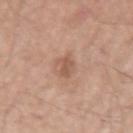Imaged during a routine full-body skin examination; the lesion was not biopsied and no histopathology is available.
A 15 mm crop from a total-body photograph taken for skin-cancer surveillance.
A male patient, aged approximately 55.
From the arm.
An algorithmic analysis of the crop reported a lesion area of about 3.5 mm² and an outline eccentricity of about 0.9 (0 = round, 1 = elongated). The software also gave a border-irregularity rating of about 4/10, a within-lesion color-variation index near 1.5/10, and a peripheral color-asymmetry measure near 0.5. It also reported a classifier nevus-likeness of about 0/100 and a detector confidence of about 100 out of 100 that the crop contains a lesion.
Captured under white-light illumination.